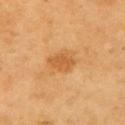• follow-up · imaged on a skin check; not biopsied
• lesion diameter · ~3.5 mm (longest diameter)
• acquisition · ~15 mm tile from a whole-body skin photo
• body site · the left upper arm
• subject · male, approximately 60 years of age